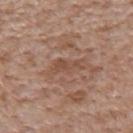workup: no biopsy performed (imaged during a skin exam); imaging modality: ~15 mm crop, total-body skin-cancer survey; tile lighting: white-light; patient: male, in their mid- to late 60s; location: the mid back; size: ~3.5 mm (longest diameter).